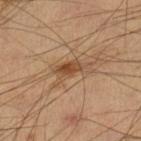  site: left lower leg
  patient:
    sex: male
    age_approx: 40
  image:
    source: total-body photography crop
    field_of_view_mm: 15